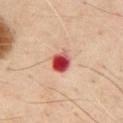Q: Was a biopsy performed?
A: total-body-photography surveillance lesion; no biopsy
Q: Patient demographics?
A: male, aged approximately 70
Q: What is the anatomic site?
A: the chest
Q: What kind of image is this?
A: ~15 mm tile from a whole-body skin photo